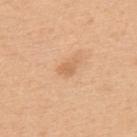Impression:
Part of a total-body skin-imaging series; this lesion was reviewed on a skin check and was not flagged for biopsy.